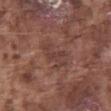workup: no biopsy performed (imaged during a skin exam) | imaging modality: 15 mm crop, total-body photography | site: the abdomen | lesion diameter: ~4.5 mm (longest diameter) | automated metrics: a shape eccentricity near 0.85 and a shape-asymmetry score of about 0.35 (0 = symmetric); a border-irregularity rating of about 4.5/10, a color-variation rating of about 2.5/10, and radial color variation of about 0.5 | tile lighting: white-light illumination | patient: male, approximately 75 years of age.